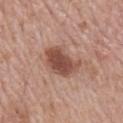biopsy status = imaged on a skin check; not biopsied